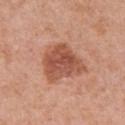biopsy_status: not biopsied; imaged during a skin examination
image:
  source: total-body photography crop
  field_of_view_mm: 15
site: left upper arm
lesion_size:
  long_diameter_mm_approx: 5.0
automated_metrics:
  area_mm2_approx: 18.0
  eccentricity: 0.5
  shape_asymmetry: 0.35
  vs_skin_darker_L: 12.0
  nevus_likeness_0_100: 20
lighting: white-light
patient:
  sex: female
  age_approx: 60